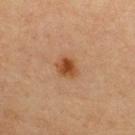{"biopsy_status": "not biopsied; imaged during a skin examination", "site": "upper back", "patient": {"sex": "female", "age_approx": 65}, "lesion_size": {"long_diameter_mm_approx": 3.0}, "image": {"source": "total-body photography crop", "field_of_view_mm": 15}, "lighting": "cross-polarized", "automated_metrics": {"area_mm2_approx": 5.5, "shape_asymmetry": 0.2}}